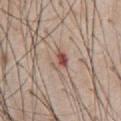anatomic site: the abdomen | illumination: white-light | image: ~15 mm tile from a whole-body skin photo | subject: male, roughly 60 years of age | automated lesion analysis: a lesion area of about 4 mm²; a nevus-likeness score of about 10/100.